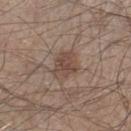<case>
  <biopsy_status>not biopsied; imaged during a skin examination</biopsy_status>
  <image>
    <source>total-body photography crop</source>
    <field_of_view_mm>15</field_of_view_mm>
  </image>
  <lesion_size>
    <long_diameter_mm_approx>4.0</long_diameter_mm_approx>
  </lesion_size>
  <site>leg</site>
  <patient>
    <sex>male</sex>
    <age_approx>45</age_approx>
  </patient>
  <automated_metrics>
    <border_irregularity_0_10>2.5</border_irregularity_0_10>
    <color_variation_0_10>3.0</color_variation_0_10>
    <peripheral_color_asymmetry>1.0</peripheral_color_asymmetry>
  </automated_metrics>
</case>